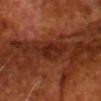Impression: Part of a total-body skin-imaging series; this lesion was reviewed on a skin check and was not flagged for biopsy. Acquisition and patient details: The patient is a male aged 78–82. This is a cross-polarized tile. Measured at roughly 5 mm in maximum diameter. The lesion is on the head or neck. This image is a 15 mm lesion crop taken from a total-body photograph.